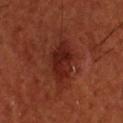The lesion was tiled from a total-body skin photograph and was not biopsied. The lesion is on the head or neck. This image is a 15 mm lesion crop taken from a total-body photograph. A male subject, approximately 55 years of age.